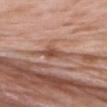Q: Is there a histopathology result?
A: catalogued during a skin exam; not biopsied
Q: What did automated image analysis measure?
A: a lesion area of about 4 mm²; an average lesion color of about L≈50 a*≈23 b*≈28 (CIELAB); an automated nevus-likeness rating near 0 out of 100 and lesion-presence confidence of about 80/100
Q: What kind of image is this?
A: ~15 mm crop, total-body skin-cancer survey
Q: What lighting was used for the tile?
A: white-light
Q: How large is the lesion?
A: ≈2.5 mm
Q: Patient demographics?
A: male, aged 58 to 62
Q: What is the anatomic site?
A: the upper back